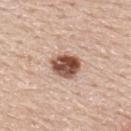biopsy_status: not biopsied; imaged during a skin examination
site: upper back
image:
  source: total-body photography crop
  field_of_view_mm: 15
patient:
  sex: male
  age_approx: 60
lesion_size:
  long_diameter_mm_approx: 3.5